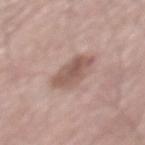The lesion was photographed on a routine skin check and not biopsied; there is no pathology result.